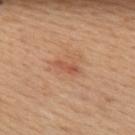follow-up: catalogued during a skin exam; not biopsied | subject: female, about 30 years old | acquisition: 15 mm crop, total-body photography | body site: the upper back | lighting: white-light illumination | diameter: ~3 mm (longest diameter).